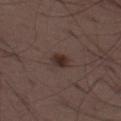Q: How large is the lesion?
A: ≈2.5 mm
Q: What is the imaging modality?
A: ~15 mm crop, total-body skin-cancer survey
Q: What lighting was used for the tile?
A: white-light
Q: Lesion location?
A: the leg
Q: Who is the patient?
A: male, aged 48–52